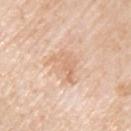Findings:
– follow-up · catalogued during a skin exam; not biopsied
– size · about 3.5 mm
– anatomic site · the left upper arm
– image-analysis metrics · a footprint of about 8.5 mm², an eccentricity of roughly 0.5, and a shape-asymmetry score of about 0.45 (0 = symmetric)
– lighting · white-light
– patient · male, aged around 80
– acquisition · ~15 mm crop, total-body skin-cancer survey